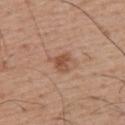Impression:
This lesion was catalogued during total-body skin photography and was not selected for biopsy.
Clinical summary:
Cropped from a total-body skin-imaging series; the visible field is about 15 mm. The subject is a male approximately 55 years of age. From the upper back.